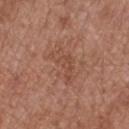| field | value |
|---|---|
| notes | no biopsy performed (imaged during a skin exam) |
| subject | female, roughly 50 years of age |
| TBP lesion metrics | a border-irregularity index near 6/10, a color-variation rating of about 2.5/10, and peripheral color asymmetry of about 1; a nevus-likeness score of about 0/100 and a detector confidence of about 100 out of 100 that the crop contains a lesion |
| image source | total-body-photography crop, ~15 mm field of view |
| lighting | white-light |
| lesion size | ~5 mm (longest diameter) |
| site | the right forearm |